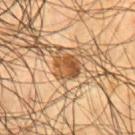Q: Is there a histopathology result?
A: imaged on a skin check; not biopsied
Q: What kind of image is this?
A: total-body-photography crop, ~15 mm field of view
Q: Who is the patient?
A: male, approximately 55 years of age
Q: What lighting was used for the tile?
A: cross-polarized illumination
Q: What did automated image analysis measure?
A: a shape eccentricity near 0.35 and a symmetry-axis asymmetry near 0.2; a border-irregularity index near 2/10 and a within-lesion color-variation index near 6.5/10; a classifier nevus-likeness of about 85/100 and a lesion-detection confidence of about 95/100
Q: Where on the body is the lesion?
A: the chest